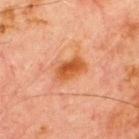• follow-up — imaged on a skin check; not biopsied
• patient — male, in their 70s
• site — the chest
• imaging modality — 15 mm crop, total-body photography